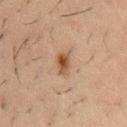anatomic site=the chest | acquisition=15 mm crop, total-body photography | subject=male, roughly 50 years of age.